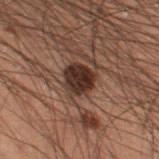– notes · no biopsy performed (imaged during a skin exam)
– anatomic site · the left thigh
– automated lesion analysis · a border-irregularity index near 2/10, a color-variation rating of about 3/10, and a peripheral color-asymmetry measure near 1
– image · 15 mm crop, total-body photography
– size · ≈3.5 mm
– illumination · cross-polarized illumination
– subject · male, aged around 55